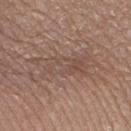notes: imaged on a skin check; not biopsied | imaging modality: 15 mm crop, total-body photography | site: the left lower leg | subject: female, in their mid- to late 50s | automated metrics: a lesion color around L≈49 a*≈17 b*≈24 in CIELAB, roughly 6 lightness units darker than nearby skin, and a normalized border contrast of about 5 | size: about 8 mm.